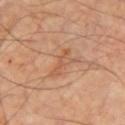workup: no biopsy performed (imaged during a skin exam)
TBP lesion metrics: a symmetry-axis asymmetry near 0.65; about 7 CIELAB-L* units darker than the surrounding skin; border irregularity of about 8.5 on a 0–10 scale and peripheral color asymmetry of about 1
body site: the chest
subject: male, aged around 60
size: ~4 mm (longest diameter)
image: total-body-photography crop, ~15 mm field of view
tile lighting: cross-polarized